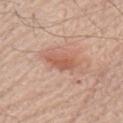notes: imaged on a skin check; not biopsied.